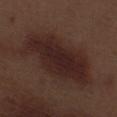Imaged during a routine full-body skin examination; the lesion was not biopsied and no histopathology is available.
A lesion tile, about 15 mm wide, cut from a 3D total-body photograph.
About 10.5 mm across.
The total-body-photography lesion software estimated a footprint of about 37 mm², an outline eccentricity of about 0.9 (0 = round, 1 = elongated), and a symmetry-axis asymmetry near 0.25. And it measured about 8 CIELAB-L* units darker than the surrounding skin and a lesion-to-skin contrast of about 9.5 (normalized; higher = more distinct). The software also gave an automated nevus-likeness rating near 35 out of 100 and lesion-presence confidence of about 100/100.
This is a white-light tile.
A male subject aged approximately 70.
On the leg.